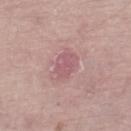biopsy_status: not biopsied; imaged during a skin examination
patient:
  sex: female
  age_approx: 70
image:
  source: total-body photography crop
  field_of_view_mm: 15
site: right lower leg